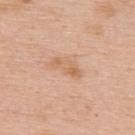No biopsy was performed on this lesion — it was imaged during a full skin examination and was not determined to be concerning. On the upper back. A region of skin cropped from a whole-body photographic capture, roughly 15 mm wide. A male patient, aged 58–62.